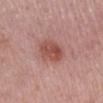Assessment:
The lesion was photographed on a routine skin check and not biopsied; there is no pathology result.
Acquisition and patient details:
The subject is a female aged approximately 50. A lesion tile, about 15 mm wide, cut from a 3D total-body photograph. Located on the leg. Captured under white-light illumination. Measured at roughly 4 mm in maximum diameter.